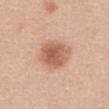A female patient, in their mid-40s. An algorithmic analysis of the crop reported an area of roughly 14 mm² and a shape eccentricity near 0.55. A 15 mm close-up extracted from a 3D total-body photography capture. The recorded lesion diameter is about 4.5 mm. On the front of the torso. Captured under white-light illumination.